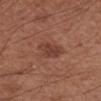Clinical impression:
This lesion was catalogued during total-body skin photography and was not selected for biopsy.